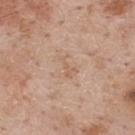Q: Was this lesion biopsied?
A: imaged on a skin check; not biopsied